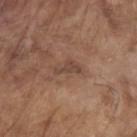patient:
  sex: male
  age_approx: 80
automated_metrics:
  shape_asymmetry: 0.55
  vs_skin_darker_L: 8.0
  vs_skin_contrast_norm: 6.5
site: left upper arm
lighting: white-light
image:
  source: total-body photography crop
  field_of_view_mm: 15
lesion_size:
  long_diameter_mm_approx: 3.0